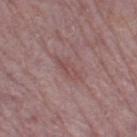Notes:
• workup — no biopsy performed (imaged during a skin exam)
• size — about 4 mm
• image-analysis metrics — a shape-asymmetry score of about 0.3 (0 = symmetric)
• imaging modality — total-body-photography crop, ~15 mm field of view
• subject — male, aged around 75
• site — the leg